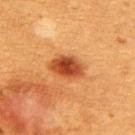Q: Was this lesion biopsied?
A: no biopsy performed (imaged during a skin exam)
Q: Where on the body is the lesion?
A: the upper back
Q: Who is the patient?
A: female, in their mid- to late 50s
Q: Automated lesion metrics?
A: an average lesion color of about L≈41 a*≈29 b*≈38 (CIELAB), a lesion–skin lightness drop of about 14, and a normalized lesion–skin contrast near 10.5; a border-irregularity index near 2/10, a color-variation rating of about 5/10, and radial color variation of about 1.5
Q: What lighting was used for the tile?
A: cross-polarized illumination
Q: What kind of image is this?
A: ~15 mm tile from a whole-body skin photo